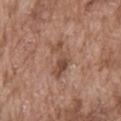Assessment: Captured during whole-body skin photography for melanoma surveillance; the lesion was not biopsied. Clinical summary: A close-up tile cropped from a whole-body skin photograph, about 15 mm across. The lesion's longest dimension is about 5 mm. A male subject, aged approximately 75. This is a white-light tile. Located on the abdomen.